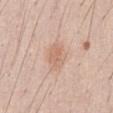No biopsy was performed on this lesion — it was imaged during a full skin examination and was not determined to be concerning. A roughly 15 mm field-of-view crop from a total-body skin photograph. Approximately 3.5 mm at its widest. The tile uses white-light illumination. A male patient aged around 35. On the abdomen.